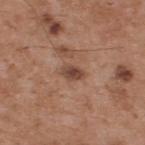Impression:
Captured during whole-body skin photography for melanoma surveillance; the lesion was not biopsied.
Clinical summary:
A male subject aged 53–57. On the upper back. Captured under white-light illumination. A close-up tile cropped from a whole-body skin photograph, about 15 mm across.